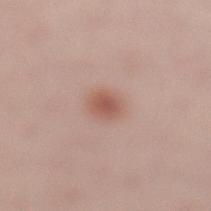Q: Is there a histopathology result?
A: imaged on a skin check; not biopsied
Q: What lighting was used for the tile?
A: white-light
Q: Where on the body is the lesion?
A: the left lower leg
Q: What is the imaging modality?
A: ~15 mm crop, total-body skin-cancer survey
Q: Lesion size?
A: ≈2.5 mm
Q: What are the patient's age and sex?
A: female, about 25 years old